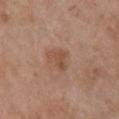biopsy status: catalogued during a skin exam; not biopsied | patient: female, about 65 years old | anatomic site: the chest | image source: ~15 mm tile from a whole-body skin photo | image-analysis metrics: an average lesion color of about L≈50 a*≈21 b*≈30 (CIELAB) and a lesion–skin lightness drop of about 7; border irregularity of about 5 on a 0–10 scale, a color-variation rating of about 2/10, and peripheral color asymmetry of about 0.5; a detector confidence of about 100 out of 100 that the crop contains a lesion | tile lighting: white-light illumination | size: ~3 mm (longest diameter).